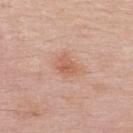biopsy_status: not biopsied; imaged during a skin examination
site: upper back
lighting: white-light
patient:
  sex: male
  age_approx: 50
lesion_size:
  long_diameter_mm_approx: 3.0
automated_metrics:
  cielab_L: 60
  cielab_a: 23
  cielab_b: 31
  vs_skin_contrast_norm: 6.0
  color_variation_0_10: 3.0
  peripheral_color_asymmetry: 1.0
  nevus_likeness_0_100: 55
  lesion_detection_confidence_0_100: 100
image:
  source: total-body photography crop
  field_of_view_mm: 15